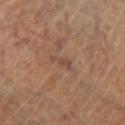Q: Was a biopsy performed?
A: catalogued during a skin exam; not biopsied
Q: Where on the body is the lesion?
A: the left lower leg
Q: What is the imaging modality?
A: ~15 mm crop, total-body skin-cancer survey
Q: How was the tile lit?
A: cross-polarized
Q: What are the patient's age and sex?
A: female, aged around 65
Q: Lesion size?
A: ~3 mm (longest diameter)